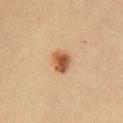Impression: The lesion was tiled from a total-body skin photograph and was not biopsied. Background: A region of skin cropped from a whole-body photographic capture, roughly 15 mm wide. A female patient roughly 35 years of age. Measured at roughly 3 mm in maximum diameter. The lesion is on the front of the torso. The total-body-photography lesion software estimated an automated nevus-likeness rating near 100 out of 100.